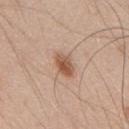Q: Was a biopsy performed?
A: catalogued during a skin exam; not biopsied
Q: Lesion location?
A: the upper back
Q: What kind of image is this?
A: ~15 mm tile from a whole-body skin photo
Q: How was the tile lit?
A: white-light
Q: What are the patient's age and sex?
A: male, approximately 50 years of age